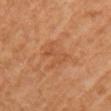Assessment:
Captured during whole-body skin photography for melanoma surveillance; the lesion was not biopsied.
Context:
About 4 mm across. This is a cross-polarized tile. The lesion is on the right upper arm. The lesion-visualizer software estimated an area of roughly 5 mm² and an eccentricity of roughly 0.9. It also reported a border-irregularity rating of about 6.5/10 and a within-lesion color-variation index near 2/10. It also reported a nevus-likeness score of about 0/100. A female subject aged approximately 60. A 15 mm close-up extracted from a 3D total-body photography capture.